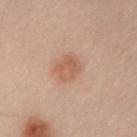workup: no biopsy performed (imaged during a skin exam) | patient: male, aged approximately 50 | acquisition: 15 mm crop, total-body photography | lighting: cross-polarized | anatomic site: the left lower leg | lesion diameter: ≈3 mm.